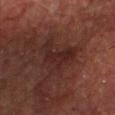Impression: This lesion was catalogued during total-body skin photography and was not selected for biopsy. Acquisition and patient details: The lesion is located on the chest. Captured under cross-polarized illumination. Automated tile analysis of the lesion measured a footprint of about 13 mm² and an outline eccentricity of about 0.5 (0 = round, 1 = elongated). The analysis additionally found an average lesion color of about L≈22 a*≈18 b*≈19 (CIELAB) and a lesion–skin lightness drop of about 6. The software also gave a border-irregularity rating of about 7/10. Cropped from a whole-body photographic skin survey; the tile spans about 15 mm. Approximately 5.5 mm at its widest. A male subject, aged 63 to 67.